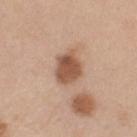<record>
  <biopsy_status>not biopsied; imaged during a skin examination</biopsy_status>
  <patient>
    <sex>male</sex>
    <age_approx>70</age_approx>
  </patient>
  <image>
    <source>total-body photography crop</source>
    <field_of_view_mm>15</field_of_view_mm>
  </image>
  <site>left upper arm</site>
</record>